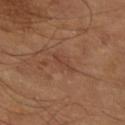Recorded during total-body skin imaging; not selected for excision or biopsy. Imaged with cross-polarized lighting. The subject is a male in their mid- to late 60s. About 3 mm across. The lesion is on the right forearm. A 15 mm crop from a total-body photograph taken for skin-cancer surveillance.